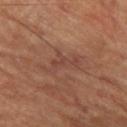Recorded during total-body skin imaging; not selected for excision or biopsy.
A region of skin cropped from a whole-body photographic capture, roughly 15 mm wide.
A male patient aged around 60.
The lesion is on the left thigh.
The tile uses cross-polarized illumination.